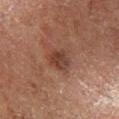A close-up tile cropped from a whole-body skin photograph, about 15 mm across. A male patient aged around 80. The total-body-photography lesion software estimated a normalized border contrast of about 7.5. The software also gave a border-irregularity index near 2/10, internal color variation of about 3 on a 0–10 scale, and a peripheral color-asymmetry measure near 1. It also reported a classifier nevus-likeness of about 5/100. The lesion is on the right lower leg. The lesion's longest dimension is about 3.5 mm.